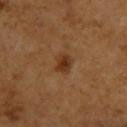Clinical impression: Captured during whole-body skin photography for melanoma surveillance; the lesion was not biopsied. Image and clinical context: A female patient about 55 years old. A roughly 15 mm field-of-view crop from a total-body skin photograph. The tile uses cross-polarized illumination. Measured at roughly 2.5 mm in maximum diameter. The lesion is located on the upper back.